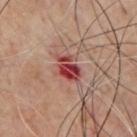Q: Was this lesion biopsied?
A: total-body-photography surveillance lesion; no biopsy
Q: How was the tile lit?
A: cross-polarized
Q: What is the imaging modality?
A: total-body-photography crop, ~15 mm field of view
Q: What is the lesion's diameter?
A: ~3.5 mm (longest diameter)
Q: Who is the patient?
A: male, aged 53 to 57
Q: What did automated image analysis measure?
A: a lesion area of about 6 mm², an outline eccentricity of about 0.75 (0 = round, 1 = elongated), and two-axis asymmetry of about 0.25; an average lesion color of about L≈43 a*≈35 b*≈25 (CIELAB), about 15 CIELAB-L* units darker than the surrounding skin, and a normalized lesion–skin contrast near 11; an automated nevus-likeness rating near 0 out of 100
Q: What is the anatomic site?
A: the chest